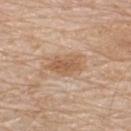Findings:
– illumination: white-light illumination
– body site: the upper back
– image: total-body-photography crop, ~15 mm field of view
– lesion diameter: ~4.5 mm (longest diameter)
– patient: male, in their 80s
– image-analysis metrics: a lesion color around L≈58 a*≈19 b*≈33 in CIELAB, roughly 10 lightness units darker than nearby skin, and a lesion-to-skin contrast of about 7 (normalized; higher = more distinct); a border-irregularity index near 3/10 and radial color variation of about 1; lesion-presence confidence of about 100/100